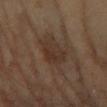biopsy status = catalogued during a skin exam; not biopsied
anatomic site = the right forearm
image source = ~15 mm tile from a whole-body skin photo
lighting = cross-polarized illumination
patient = female, aged around 70
image-analysis metrics = a classifier nevus-likeness of about 0/100
diameter = ≈3.5 mm